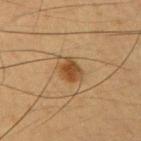notes = total-body-photography surveillance lesion; no biopsy | subject = male, approximately 55 years of age | tile lighting = cross-polarized | location = the left upper arm | acquisition = total-body-photography crop, ~15 mm field of view | lesion size = ~3 mm (longest diameter) | automated metrics = an eccentricity of roughly 0.55; border irregularity of about 2 on a 0–10 scale, a within-lesion color-variation index near 3/10, and peripheral color asymmetry of about 1; an automated nevus-likeness rating near 95 out of 100 and a lesion-detection confidence of about 100/100.